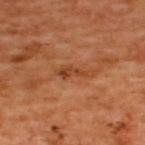biopsy_status: not biopsied; imaged during a skin examination
lighting: cross-polarized
patient:
  sex: male
  age_approx: 50
image:
  source: total-body photography crop
  field_of_view_mm: 15
lesion_size:
  long_diameter_mm_approx: 4.0
site: upper back